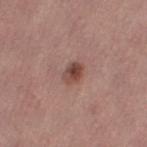Notes:
• notes · catalogued during a skin exam; not biopsied
• subject · female, aged approximately 50
• site · the right thigh
• image source · ~15 mm crop, total-body skin-cancer survey
• lesion size · ≈2.5 mm
• illumination · white-light